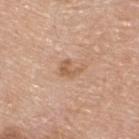biopsy status = no biopsy performed (imaged during a skin exam)
acquisition = ~15 mm tile from a whole-body skin photo
subject = male, about 80 years old
diameter = ≈2.5 mm
body site = the upper back
image-analysis metrics = an area of roughly 3.5 mm² and two-axis asymmetry of about 0.45; a mean CIELAB color near L≈59 a*≈20 b*≈33; a nevus-likeness score of about 0/100 and a lesion-detection confidence of about 100/100
lighting = white-light